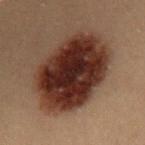follow-up: total-body-photography surveillance lesion; no biopsy | automated lesion analysis: a mean CIELAB color near L≈22 a*≈17 b*≈20, about 16 CIELAB-L* units darker than the surrounding skin, and a lesion-to-skin contrast of about 16.5 (normalized; higher = more distinct) | patient: female, approximately 20 years of age | location: the mid back | lighting: cross-polarized illumination | lesion diameter: ~9 mm (longest diameter) | image source: 15 mm crop, total-body photography.